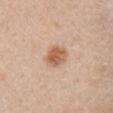Clinical impression: The lesion was photographed on a routine skin check and not biopsied; there is no pathology result. Acquisition and patient details: Automated image analysis of the tile measured a footprint of about 6 mm², an eccentricity of roughly 0.25, and two-axis asymmetry of about 0.15. It also reported a lesion color around L≈59 a*≈21 b*≈33 in CIELAB, a lesion–skin lightness drop of about 12, and a normalized border contrast of about 8.5. It also reported a nevus-likeness score of about 95/100 and a lesion-detection confidence of about 100/100. The recorded lesion diameter is about 2.5 mm. Cropped from a whole-body photographic skin survey; the tile spans about 15 mm. The tile uses white-light illumination. On the chest. A female subject in their 30s.